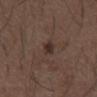workup = total-body-photography surveillance lesion; no biopsy | tile lighting = white-light illumination | lesion diameter = ~2.5 mm (longest diameter) | anatomic site = the abdomen | image-analysis metrics = a footprint of about 3.5 mm², an eccentricity of roughly 0.75, and a symmetry-axis asymmetry near 0.35; a peripheral color-asymmetry measure near 1; an automated nevus-likeness rating near 55 out of 100 and lesion-presence confidence of about 100/100 | patient = male, in their 50s | image source = total-body-photography crop, ~15 mm field of view.